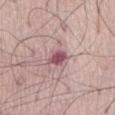Impression:
No biopsy was performed on this lesion — it was imaged during a full skin examination and was not determined to be concerning.
Clinical summary:
Captured under white-light illumination. The lesion's longest dimension is about 2.5 mm. A male subject, aged approximately 80. Automated tile analysis of the lesion measured a lesion area of about 4.5 mm² and an eccentricity of roughly 0.65. The software also gave a lesion color around L≈52 a*≈25 b*≈15 in CIELAB, a lesion–skin lightness drop of about 14, and a normalized border contrast of about 10. A close-up tile cropped from a whole-body skin photograph, about 15 mm across. The lesion is located on the abdomen.